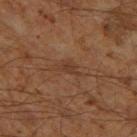{"biopsy_status": "not biopsied; imaged during a skin examination", "image": {"source": "total-body photography crop", "field_of_view_mm": 15}, "lesion_size": {"long_diameter_mm_approx": 3.5}, "site": "left thigh", "lighting": "cross-polarized", "patient": {"sex": "male", "age_approx": 60}}